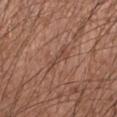follow-up=imaged on a skin check; not biopsied
lesion size=about 3.5 mm
automated lesion analysis=a footprint of about 5 mm², a shape eccentricity near 0.9, and a symmetry-axis asymmetry near 0.3; border irregularity of about 4 on a 0–10 scale, a within-lesion color-variation index near 3/10, and radial color variation of about 1; a detector confidence of about 60 out of 100 that the crop contains a lesion
subject=male, roughly 30 years of age
image source=total-body-photography crop, ~15 mm field of view
body site=the left forearm